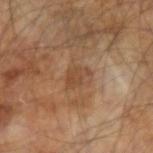A male patient, aged around 65. Longest diameter approximately 3 mm. A region of skin cropped from a whole-body photographic capture, roughly 15 mm wide. Automated tile analysis of the lesion measured a lesion color around L≈44 a*≈18 b*≈31 in CIELAB, a lesion–skin lightness drop of about 7, and a lesion-to-skin contrast of about 5.5 (normalized; higher = more distinct). The software also gave a classifier nevus-likeness of about 0/100. On the left forearm. Captured under cross-polarized illumination.